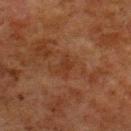Part of a total-body skin-imaging series; this lesion was reviewed on a skin check and was not flagged for biopsy. The total-body-photography lesion software estimated a lesion area of about 3 mm², an outline eccentricity of about 0.85 (0 = round, 1 = elongated), and two-axis asymmetry of about 0.45. The software also gave a mean CIELAB color near L≈27 a*≈19 b*≈26 and a lesion-to-skin contrast of about 6 (normalized; higher = more distinct). And it measured border irregularity of about 4.5 on a 0–10 scale and peripheral color asymmetry of about 0. A male subject approximately 80 years of age. Cropped from a whole-body photographic skin survey; the tile spans about 15 mm. On the upper back. Longest diameter approximately 2.5 mm.